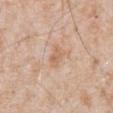The lesion was photographed on a routine skin check and not biopsied; there is no pathology result. Captured under white-light illumination. On the front of the torso. The subject is a male about 65 years old. Measured at roughly 2.5 mm in maximum diameter. A 15 mm close-up extracted from a 3D total-body photography capture.